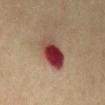Captured during whole-body skin photography for melanoma surveillance; the lesion was not biopsied.
From the back.
The lesion-visualizer software estimated a mean CIELAB color near L≈34 a*≈24 b*≈21, roughly 16 lightness units darker than nearby skin, and a normalized border contrast of about 13.5. It also reported a border-irregularity index near 1.5/10, a color-variation rating of about 10/10, and peripheral color asymmetry of about 4.5. It also reported a classifier nevus-likeness of about 0/100.
A male patient aged approximately 75.
A 15 mm close-up extracted from a 3D total-body photography capture.
The lesion's longest dimension is about 4.5 mm.